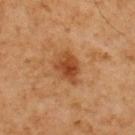<case>
  <biopsy_status>not biopsied; imaged during a skin examination</biopsy_status>
  <automated_metrics>
    <eccentricity>0.65</eccentricity>
    <shape_asymmetry>0.25</shape_asymmetry>
    <nevus_likeness_0_100>85</nevus_likeness_0_100>
    <lesion_detection_confidence_0_100>100</lesion_detection_confidence_0_100>
  </automated_metrics>
  <lighting>cross-polarized</lighting>
  <site>upper back</site>
  <patient>
    <sex>male</sex>
    <age_approx>60</age_approx>
  </patient>
  <lesion_size>
    <long_diameter_mm_approx>3.5</long_diameter_mm_approx>
  </lesion_size>
  <image>
    <source>total-body photography crop</source>
    <field_of_view_mm>15</field_of_view_mm>
  </image>
</case>